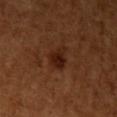Acquisition and patient details: A female subject, aged 53–57. A close-up tile cropped from a whole-body skin photograph, about 15 mm across. On the left upper arm. Captured under cross-polarized illumination.